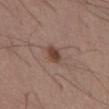The lesion is located on the front of the torso.
This image is a 15 mm lesion crop taken from a total-body photograph.
The lesion's longest dimension is about 2.5 mm.
The tile uses white-light illumination.
The lesion-visualizer software estimated an outline eccentricity of about 0.65 (0 = round, 1 = elongated) and a symmetry-axis asymmetry near 0.3. The software also gave an automated nevus-likeness rating near 95 out of 100 and a detector confidence of about 100 out of 100 that the crop contains a lesion.
A male patient aged 53 to 57.